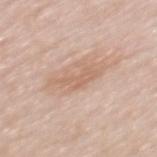Clinical impression:
No biopsy was performed on this lesion — it was imaged during a full skin examination and was not determined to be concerning.
Context:
Imaged with white-light lighting. On the mid back. The lesion-visualizer software estimated a footprint of about 6.5 mm², a shape eccentricity near 0.9, and a shape-asymmetry score of about 0.5 (0 = symmetric). The analysis additionally found a classifier nevus-likeness of about 0/100 and lesion-presence confidence of about 100/100. The recorded lesion diameter is about 4.5 mm. A female subject about 60 years old. Cropped from a total-body skin-imaging series; the visible field is about 15 mm.